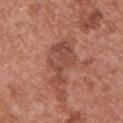biopsy status: no biopsy performed (imaged during a skin exam).